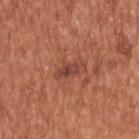Assessment: The lesion was tiled from a total-body skin photograph and was not biopsied. Clinical summary: The patient is a male aged approximately 65. The recorded lesion diameter is about 3 mm. Located on the upper back. Imaged with white-light lighting. A 15 mm close-up extracted from a 3D total-body photography capture. The lesion-visualizer software estimated a footprint of about 3.5 mm² and a symmetry-axis asymmetry near 0.3. It also reported border irregularity of about 3.5 on a 0–10 scale and a peripheral color-asymmetry measure near 2. The analysis additionally found a classifier nevus-likeness of about 0/100 and a detector confidence of about 100 out of 100 that the crop contains a lesion.